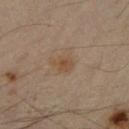Captured during whole-body skin photography for melanoma surveillance; the lesion was not biopsied.
The total-body-photography lesion software estimated roughly 6 lightness units darker than nearby skin and a normalized lesion–skin contrast near 6. And it measured a peripheral color-asymmetry measure near 0.5.
Measured at roughly 2.5 mm in maximum diameter.
A male subject aged approximately 45.
A 15 mm close-up tile from a total-body photography series done for melanoma screening.
On the right forearm.
Captured under cross-polarized illumination.